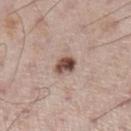{"biopsy_status": "not biopsied; imaged during a skin examination", "patient": {"sex": "male", "age_approx": 60}, "image": {"source": "total-body photography crop", "field_of_view_mm": 15}, "lesion_size": {"long_diameter_mm_approx": 2.5}, "lighting": "white-light", "site": "leg"}